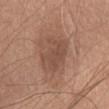Notes:
• follow-up: total-body-photography surveillance lesion; no biopsy
• imaging modality: ~15 mm crop, total-body skin-cancer survey
• diameter: about 4.5 mm
• illumination: white-light
• body site: the front of the torso
• patient: male, approximately 65 years of age